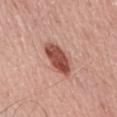Background: About 5 mm across. A male subject in their mid- to late 60s. The lesion is on the chest. Imaged with white-light lighting. Cropped from a whole-body photographic skin survey; the tile spans about 15 mm.